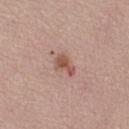The lesion was tiled from a total-body skin photograph and was not biopsied.
On the right thigh.
A female subject roughly 50 years of age.
Approximately 3 mm at its widest.
A roughly 15 mm field-of-view crop from a total-body skin photograph.
This is a white-light tile.